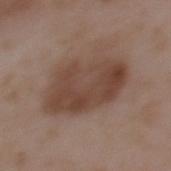{
  "biopsy_status": "not biopsied; imaged during a skin examination",
  "lesion_size": {
    "long_diameter_mm_approx": 7.5
  },
  "image": {
    "source": "total-body photography crop",
    "field_of_view_mm": 15
  },
  "patient": {
    "sex": "male",
    "age_approx": 50
  },
  "lighting": "white-light",
  "automated_metrics": {
    "area_mm2_approx": 29.0,
    "eccentricity": 0.8,
    "shape_asymmetry": 0.2,
    "border_irregularity_0_10": 2.5,
    "color_variation_0_10": 4.5,
    "peripheral_color_asymmetry": 1.5
  },
  "site": "back"
}